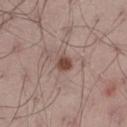Case summary:
• biopsy status — total-body-photography surveillance lesion; no biopsy
• acquisition — total-body-photography crop, ~15 mm field of view
• body site — the left thigh
• lighting — white-light illumination
• patient — male, aged around 70